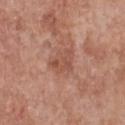Recorded during total-body skin imaging; not selected for excision or biopsy. This is a white-light tile. A female patient in their 60s. Cropped from a whole-body photographic skin survey; the tile spans about 15 mm. From the chest.